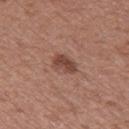This image is a 15 mm lesion crop taken from a total-body photograph. Located on the left thigh. A female subject aged around 40. An algorithmic analysis of the crop reported a lesion-detection confidence of about 100/100. The lesion's longest dimension is about 3 mm.